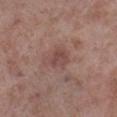No biopsy was performed on this lesion — it was imaged during a full skin examination and was not determined to be concerning. Imaged with white-light lighting. Located on the right lower leg. The total-body-photography lesion software estimated a footprint of about 5 mm² and an outline eccentricity of about 0.55 (0 = round, 1 = elongated). And it measured a classifier nevus-likeness of about 0/100 and a detector confidence of about 100 out of 100 that the crop contains a lesion. A male patient, roughly 55 years of age. A 15 mm close-up tile from a total-body photography series done for melanoma screening. The recorded lesion diameter is about 2.5 mm.